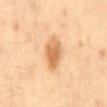* biopsy status — imaged on a skin check; not biopsied
* image — 15 mm crop, total-body photography
* body site — the back
* subject — male, aged around 40
* TBP lesion metrics — a border-irregularity index near 1.5/10 and peripheral color asymmetry of about 1.5; a detector confidence of about 100 out of 100 that the crop contains a lesion
* lighting — cross-polarized illumination
* lesion diameter — ~4 mm (longest diameter)